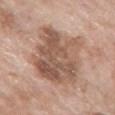{
  "biopsy_status": "not biopsied; imaged during a skin examination",
  "site": "front of the torso",
  "patient": {
    "sex": "female",
    "age_approx": 75
  },
  "lesion_size": {
    "long_diameter_mm_approx": 8.0
  },
  "lighting": "white-light",
  "image": {
    "source": "total-body photography crop",
    "field_of_view_mm": 15
  }
}